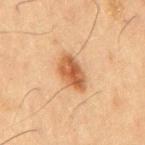Q: Is there a histopathology result?
A: imaged on a skin check; not biopsied
Q: Lesion size?
A: ~4.5 mm (longest diameter)
Q: Patient demographics?
A: male, aged approximately 60
Q: Illumination type?
A: cross-polarized illumination
Q: How was this image acquired?
A: 15 mm crop, total-body photography